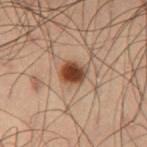Part of a total-body skin-imaging series; this lesion was reviewed on a skin check and was not flagged for biopsy.
About 3 mm across.
The lesion is on the left thigh.
Cropped from a whole-body photographic skin survey; the tile spans about 15 mm.
The patient is a male aged approximately 55.
The tile uses cross-polarized illumination.
The lesion-visualizer software estimated an average lesion color of about L≈35 a*≈18 b*≈26 (CIELAB), a lesion–skin lightness drop of about 14, and a lesion-to-skin contrast of about 12 (normalized; higher = more distinct). The analysis additionally found border irregularity of about 1.5 on a 0–10 scale. It also reported a nevus-likeness score of about 100/100 and a lesion-detection confidence of about 100/100.